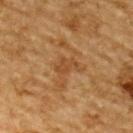Captured during whole-body skin photography for melanoma surveillance; the lesion was not biopsied.
The lesion's longest dimension is about 3 mm.
A male subject, aged 83 or older.
The lesion is located on the upper back.
A 15 mm close-up tile from a total-body photography series done for melanoma screening.
Automated image analysis of the tile measured an area of roughly 4 mm² and two-axis asymmetry of about 0.4. The analysis additionally found an average lesion color of about L≈40 a*≈19 b*≈34 (CIELAB) and a normalized border contrast of about 5.5. The software also gave a border-irregularity rating of about 5/10, a within-lesion color-variation index near 1/10, and radial color variation of about 0.5. It also reported a classifier nevus-likeness of about 0/100 and lesion-presence confidence of about 95/100.
Imaged with cross-polarized lighting.